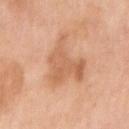Clinical impression:
This lesion was catalogued during total-body skin photography and was not selected for biopsy.
Image and clinical context:
This image is a 15 mm lesion crop taken from a total-body photograph. A female subject aged 63 to 67. On the right upper arm. Automated tile analysis of the lesion measured a footprint of about 17 mm², an eccentricity of roughly 0.6, and a shape-asymmetry score of about 0.55 (0 = symmetric). And it measured a mean CIELAB color near L≈63 a*≈23 b*≈36, about 9 CIELAB-L* units darker than the surrounding skin, and a normalized lesion–skin contrast near 6. And it measured a classifier nevus-likeness of about 0/100. Approximately 6 mm at its widest. This is a white-light tile.